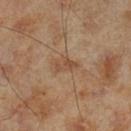Impression:
Recorded during total-body skin imaging; not selected for excision or biopsy.
Context:
About 2.5 mm across. The tile uses cross-polarized illumination. The lesion-visualizer software estimated a lesion color around L≈47 a*≈18 b*≈31 in CIELAB, about 7 CIELAB-L* units darker than the surrounding skin, and a lesion-to-skin contrast of about 6 (normalized; higher = more distinct). The software also gave a nevus-likeness score of about 0/100 and lesion-presence confidence of about 100/100. Cropped from a total-body skin-imaging series; the visible field is about 15 mm. The subject is a male approximately 70 years of age. Located on the leg.